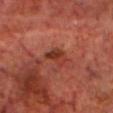follow-up = no biopsy performed (imaged during a skin exam); image = total-body-photography crop, ~15 mm field of view; body site = the chest; subject = male, aged 68–72.